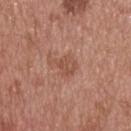tile lighting=white-light | patient=male, aged around 55 | acquisition=~15 mm crop, total-body skin-cancer survey | lesion diameter=~3.5 mm (longest diameter) | anatomic site=the upper back.